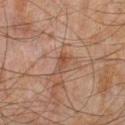{
  "biopsy_status": "not biopsied; imaged during a skin examination",
  "lesion_size": {
    "long_diameter_mm_approx": 3.0
  },
  "automated_metrics": {
    "vs_skin_darker_L": 6.0,
    "vs_skin_contrast_norm": 6.0,
    "border_irregularity_0_10": 3.5,
    "peripheral_color_asymmetry": 0.5
  },
  "patient": {
    "sex": "male",
    "age_approx": 45
  },
  "site": "left lower leg",
  "image": {
    "source": "total-body photography crop",
    "field_of_view_mm": 15
  }
}